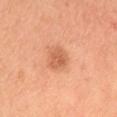The lesion was photographed on a routine skin check and not biopsied; there is no pathology result. The recorded lesion diameter is about 3 mm. Located on the head or neck. A close-up tile cropped from a whole-body skin photograph, about 15 mm across. The subject is a female aged 33–37.